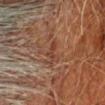Imaged during a routine full-body skin examination; the lesion was not biopsied and no histopathology is available. The total-body-photography lesion software estimated a footprint of about 3.5 mm² and a shape-asymmetry score of about 0.55 (0 = symmetric). And it measured border irregularity of about 6 on a 0–10 scale, internal color variation of about 0 on a 0–10 scale, and peripheral color asymmetry of about 0. It also reported a nevus-likeness score of about 5/100 and a lesion-detection confidence of about 75/100. On the head or neck. Captured under cross-polarized illumination. A lesion tile, about 15 mm wide, cut from a 3D total-body photograph. The subject is a male aged 78–82.